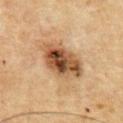Assessment: Imaged during a routine full-body skin examination; the lesion was not biopsied and no histopathology is available. Image and clinical context: The lesion is located on the chest. An algorithmic analysis of the crop reported a color-variation rating of about 10/10. Longest diameter approximately 6 mm. A male subject, in their mid-60s. This image is a 15 mm lesion crop taken from a total-body photograph. The tile uses cross-polarized illumination.